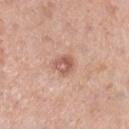This lesion was catalogued during total-body skin photography and was not selected for biopsy.
The recorded lesion diameter is about 3 mm.
The lesion is located on the right lower leg.
A region of skin cropped from a whole-body photographic capture, roughly 15 mm wide.
A female subject roughly 55 years of age.
The tile uses white-light illumination.
Automated image analysis of the tile measured a footprint of about 5 mm², a shape eccentricity near 0.55, and two-axis asymmetry of about 0.25. The analysis additionally found a border-irregularity index near 2/10, a within-lesion color-variation index near 6/10, and a peripheral color-asymmetry measure near 2.5.